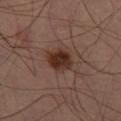Impression: Captured during whole-body skin photography for melanoma surveillance; the lesion was not biopsied.